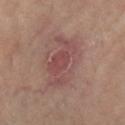biopsy status: no biopsy performed (imaged during a skin exam)
lighting: cross-polarized illumination
site: the right lower leg
automated lesion analysis: a footprint of about 16 mm² and a shape-asymmetry score of about 0.3 (0 = symmetric); an average lesion color of about L≈48 a*≈22 b*≈21 (CIELAB), roughly 8 lightness units darker than nearby skin, and a normalized border contrast of about 6; internal color variation of about 4 on a 0–10 scale and radial color variation of about 1; an automated nevus-likeness rating near 10 out of 100 and a lesion-detection confidence of about 100/100
acquisition: 15 mm crop, total-body photography
patient: female, aged approximately 65
size: about 6 mm